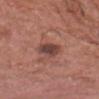Case summary:
• follow-up — imaged on a skin check; not biopsied
• lighting — white-light illumination
• site — the head or neck
• subject — male, aged 73–77
• acquisition — ~15 mm crop, total-body skin-cancer survey